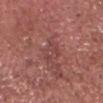Part of a total-body skin-imaging series; this lesion was reviewed on a skin check and was not flagged for biopsy. Automated tile analysis of the lesion measured a footprint of about 5 mm², an eccentricity of roughly 0.75, and a symmetry-axis asymmetry near 0.35. It also reported an average lesion color of about L≈44 a*≈26 b*≈22 (CIELAB), a lesion–skin lightness drop of about 6, and a lesion-to-skin contrast of about 5 (normalized; higher = more distinct). It also reported a detector confidence of about 85 out of 100 that the crop contains a lesion. A male patient, about 70 years old. A roughly 15 mm field-of-view crop from a total-body skin photograph. On the head or neck. Approximately 3.5 mm at its widest.